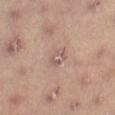image source: 15 mm crop, total-body photography; body site: the right thigh; lesion diameter: about 3 mm; subject: male, about 55 years old; illumination: cross-polarized illumination.